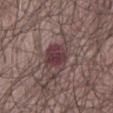Findings:
- follow-up — catalogued during a skin exam; not biopsied
- size — ≈4 mm
- tile lighting — white-light
- imaging modality — ~15 mm tile from a whole-body skin photo
- patient — male, in their 80s
- anatomic site — the abdomen